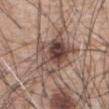{"biopsy_status": "not biopsied; imaged during a skin examination", "image": {"source": "total-body photography crop", "field_of_view_mm": 15}, "lesion_size": {"long_diameter_mm_approx": 5.5}, "patient": {"sex": "male", "age_approx": 60}, "lighting": "white-light", "site": "abdomen"}